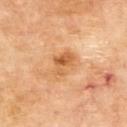Part of a total-body skin-imaging series; this lesion was reviewed on a skin check and was not flagged for biopsy. Approximately 3.5 mm at its widest. A region of skin cropped from a whole-body photographic capture, roughly 15 mm wide. A male patient, in their 70s. The lesion is located on the upper back. Automated image analysis of the tile measured a lesion area of about 6 mm², an eccentricity of roughly 0.7, and a symmetry-axis asymmetry near 0.35. And it measured a lesion–skin lightness drop of about 10 and a lesion-to-skin contrast of about 7 (normalized; higher = more distinct). The software also gave a border-irregularity rating of about 4/10 and a within-lesion color-variation index near 6/10. And it measured a classifier nevus-likeness of about 0/100 and lesion-presence confidence of about 100/100.